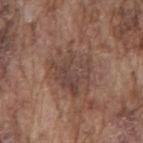Recorded during total-body skin imaging; not selected for excision or biopsy. Approximately 5.5 mm at its widest. A male subject, in their mid-70s. This is a white-light tile. A 15 mm crop from a total-body photograph taken for skin-cancer surveillance. The total-body-photography lesion software estimated a within-lesion color-variation index near 4.5/10 and a peripheral color-asymmetry measure near 1.5. The lesion is located on the mid back.